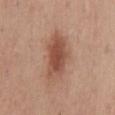No biopsy was performed on this lesion — it was imaged during a full skin examination and was not determined to be concerning. A male subject in their mid-50s. A 15 mm close-up tile from a total-body photography series done for melanoma screening. Captured under white-light illumination. An algorithmic analysis of the crop reported a lesion area of about 15 mm² and a shape-asymmetry score of about 0.25 (0 = symmetric). The software also gave an average lesion color of about L≈51 a*≈22 b*≈29 (CIELAB), a lesion–skin lightness drop of about 12, and a normalized border contrast of about 8.5. The software also gave a border-irregularity rating of about 3/10, a color-variation rating of about 4.5/10, and radial color variation of about 1. It also reported a classifier nevus-likeness of about 95/100 and a detector confidence of about 100 out of 100 that the crop contains a lesion. From the mid back. The lesion's longest dimension is about 6 mm.